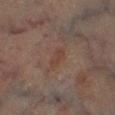notes=total-body-photography surveillance lesion; no biopsy | illumination=cross-polarized illumination | patient=male, in their 70s | image source=total-body-photography crop, ~15 mm field of view | lesion diameter=≈2.5 mm | anatomic site=the left lower leg | automated metrics=a lesion color around L≈32 a*≈16 b*≈20 in CIELAB, roughly 4 lightness units darker than nearby skin, and a normalized border contrast of about 5; a border-irregularity rating of about 4.5/10, a color-variation rating of about 0/10, and radial color variation of about 0; a nevus-likeness score of about 25/100 and lesion-presence confidence of about 100/100.